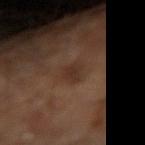Clinical impression:
The lesion was photographed on a routine skin check and not biopsied; there is no pathology result.
Acquisition and patient details:
On the right forearm. Cropped from a whole-body photographic skin survey; the tile spans about 15 mm. A male subject about 65 years old.